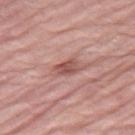A female patient roughly 70 years of age. Longest diameter approximately 3 mm. A 15 mm close-up extracted from a 3D total-body photography capture. The tile uses white-light illumination. Located on the right thigh. The lesion-visualizer software estimated an area of roughly 4.5 mm² and two-axis asymmetry of about 0.25. And it measured roughly 11 lightness units darker than nearby skin. The software also gave a detector confidence of about 100 out of 100 that the crop contains a lesion.